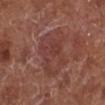This lesion was catalogued during total-body skin photography and was not selected for biopsy.
A region of skin cropped from a whole-body photographic capture, roughly 15 mm wide.
Longest diameter approximately 6 mm.
On the left lower leg.
This is a white-light tile.
A male patient, in their mid-70s.